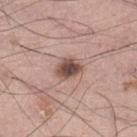Q: Was this lesion biopsied?
A: no biopsy performed (imaged during a skin exam)
Q: How large is the lesion?
A: ~3.5 mm (longest diameter)
Q: What is the anatomic site?
A: the left leg
Q: Who is the patient?
A: male, aged 68–72
Q: What is the imaging modality?
A: 15 mm crop, total-body photography
Q: Illumination type?
A: white-light illumination